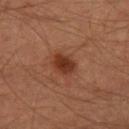Case summary:
• patient · male, aged approximately 65
• imaging modality · total-body-photography crop, ~15 mm field of view
• site · the right thigh
• lesion size · ≈3 mm